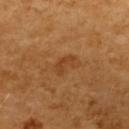notes: imaged on a skin check; not biopsied
size: ≈3 mm
TBP lesion metrics: an average lesion color of about L≈43 a*≈25 b*≈39 (CIELAB), a lesion–skin lightness drop of about 7, and a lesion-to-skin contrast of about 6 (normalized; higher = more distinct)
subject: female, aged approximately 55
anatomic site: the upper back
illumination: cross-polarized
imaging modality: total-body-photography crop, ~15 mm field of view